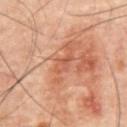{
  "biopsy_status": "not biopsied; imaged during a skin examination",
  "image": {
    "source": "total-body photography crop",
    "field_of_view_mm": 15
  },
  "site": "abdomen",
  "lesion_size": {
    "long_diameter_mm_approx": 2.5
  },
  "patient": {
    "sex": "male",
    "age_approx": 60
  },
  "lighting": "cross-polarized"
}